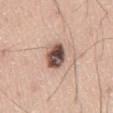Impression:
This lesion was catalogued during total-body skin photography and was not selected for biopsy.
Background:
A close-up tile cropped from a whole-body skin photograph, about 15 mm across. The lesion-visualizer software estimated a footprint of about 8 mm², an eccentricity of roughly 0.5, and two-axis asymmetry of about 0.15. The software also gave a mean CIELAB color near L≈50 a*≈18 b*≈23, about 21 CIELAB-L* units darker than the surrounding skin, and a normalized border contrast of about 13.5. The tile uses white-light illumination. Approximately 3.5 mm at its widest. A male subject, about 60 years old. The lesion is on the lower back.